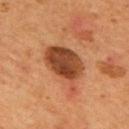workup: catalogued during a skin exam; not biopsied | illumination: cross-polarized | location: the back | image-analysis metrics: a footprint of about 17 mm² and an eccentricity of roughly 0.8; a nevus-likeness score of about 60/100 | diameter: about 6.5 mm | image: 15 mm crop, total-body photography | patient: male, aged approximately 55.